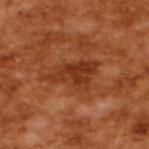Recorded during total-body skin imaging; not selected for excision or biopsy.
Cropped from a total-body skin-imaging series; the visible field is about 15 mm.
A male subject aged around 65.
About 5.5 mm across.
Imaged with cross-polarized lighting.